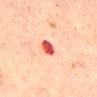notes: total-body-photography surveillance lesion; no biopsy | size: about 3 mm | acquisition: total-body-photography crop, ~15 mm field of view | body site: the mid back | lighting: cross-polarized | TBP lesion metrics: a normalized border contrast of about 11.5 | subject: male, in their mid- to late 40s.